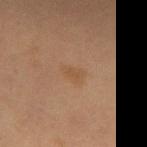notes — total-body-photography surveillance lesion; no biopsy | lesion diameter — ≈3 mm | image — ~15 mm tile from a whole-body skin photo | site — the left thigh | patient — female, in their mid- to late 50s | illumination — cross-polarized illumination | image-analysis metrics — a footprint of about 4.5 mm² and a shape eccentricity near 0.8; a border-irregularity index near 3/10 and internal color variation of about 2 on a 0–10 scale; a classifier nevus-likeness of about 0/100 and a detector confidence of about 100 out of 100 that the crop contains a lesion.